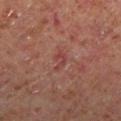  lighting: cross-polarized
  automated_metrics:
    cielab_L: 39
    cielab_a: 26
    cielab_b: 22
    vs_skin_darker_L: 6.0
    border_irregularity_0_10: 7.0
    color_variation_0_10: 0.0
    peripheral_color_asymmetry: 0.0
  patient:
    sex: male
    age_approx: 60
  site: left lower leg
  image:
    source: total-body photography crop
    field_of_view_mm: 15
  lesion_size:
    long_diameter_mm_approx: 3.0